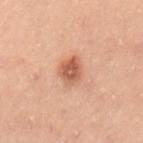{"biopsy_status": "not biopsied; imaged during a skin examination", "site": "lower back", "lesion_size": {"long_diameter_mm_approx": 3.5}, "patient": {"sex": "male", "age_approx": 50}, "image": {"source": "total-body photography crop", "field_of_view_mm": 15}, "lighting": "cross-polarized"}